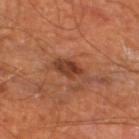Notes:
- workup — catalogued during a skin exam; not biopsied
- subject — male, aged 63 to 67
- lesion diameter — ≈4.5 mm
- tile lighting — cross-polarized illumination
- site — the left thigh
- automated metrics — a lesion area of about 6.5 mm², an outline eccentricity of about 0.9 (0 = round, 1 = elongated), and a shape-asymmetry score of about 0.25 (0 = symmetric); lesion-presence confidence of about 100/100
- acquisition — ~15 mm tile from a whole-body skin photo